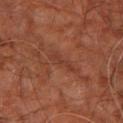biopsy status = total-body-photography surveillance lesion; no biopsy | illumination = cross-polarized | subject = male, aged 58–62 | lesion diameter = ~3.5 mm (longest diameter) | anatomic site = the right leg | imaging modality = ~15 mm tile from a whole-body skin photo.